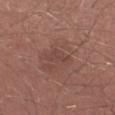follow-up — no biopsy performed (imaged during a skin exam)
location — the arm
patient — male, aged approximately 30
lesion size — ≈3.5 mm
image source — ~15 mm tile from a whole-body skin photo
illumination — white-light illumination
TBP lesion metrics — a footprint of about 5 mm², a shape eccentricity near 0.9, and a shape-asymmetry score of about 0.4 (0 = symmetric); an average lesion color of about L≈44 a*≈20 b*≈23 (CIELAB), a lesion–skin lightness drop of about 6, and a normalized lesion–skin contrast near 4.5; a nevus-likeness score of about 0/100